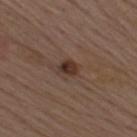The lesion was photographed on a routine skin check and not biopsied; there is no pathology result. A male patient about 70 years old. A close-up tile cropped from a whole-body skin photograph, about 15 mm across. On the right upper arm.